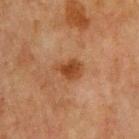{"biopsy_status": "not biopsied; imaged during a skin examination", "site": "chest", "image": {"source": "total-body photography crop", "field_of_view_mm": 15}, "patient": {"sex": "male", "age_approx": 65}}